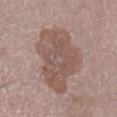A 15 mm close-up extracted from a 3D total-body photography capture. Longest diameter approximately 7.5 mm. Captured under white-light illumination. The lesion is on the leg. The subject is a male aged 68–72. Automated tile analysis of the lesion measured a footprint of about 33 mm² and an eccentricity of roughly 0.55. The analysis additionally found a border-irregularity rating of about 3.5/10, internal color variation of about 3.5 on a 0–10 scale, and a peripheral color-asymmetry measure near 1. The software also gave a classifier nevus-likeness of about 0/100.